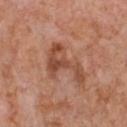No biopsy was performed on this lesion — it was imaged during a full skin examination and was not determined to be concerning. A male subject, in their mid-60s. The tile uses white-light illumination. On the chest. Measured at roughly 6 mm in maximum diameter. A 15 mm crop from a total-body photograph taken for skin-cancer surveillance.